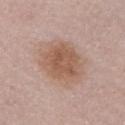Q: Is there a histopathology result?
A: imaged on a skin check; not biopsied
Q: What lighting was used for the tile?
A: white-light
Q: Lesion location?
A: the chest
Q: How large is the lesion?
A: ~5.5 mm (longest diameter)
Q: What are the patient's age and sex?
A: female, aged 48 to 52
Q: What did automated image analysis measure?
A: a lesion area of about 18 mm² and two-axis asymmetry of about 0.15; a mean CIELAB color near L≈56 a*≈19 b*≈28 and a normalized border contrast of about 7.5; internal color variation of about 3.5 on a 0–10 scale and peripheral color asymmetry of about 1
Q: How was this image acquired?
A: total-body-photography crop, ~15 mm field of view